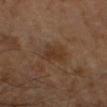Assessment: This lesion was catalogued during total-body skin photography and was not selected for biopsy. Clinical summary: On the right arm. The subject is a male about 60 years old. Longest diameter approximately 3 mm. The tile uses cross-polarized illumination. This image is a 15 mm lesion crop taken from a total-body photograph.